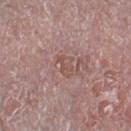<tbp_lesion>
  <site>left thigh</site>
  <patient>
    <sex>male</sex>
    <age_approx>80</age_approx>
  </patient>
  <image>
    <source>total-body photography crop</source>
    <field_of_view_mm>15</field_of_view_mm>
  </image>
  <lesion_size>
    <long_diameter_mm_approx>2.5</long_diameter_mm_approx>
  </lesion_size>
  <automated_metrics>
    <color_variation_0_10>2.0</color_variation_0_10>
    <peripheral_color_asymmetry>0.5</peripheral_color_asymmetry>
    <nevus_likeness_0_100>0</nevus_likeness_0_100>
  </automated_metrics>
</tbp_lesion>